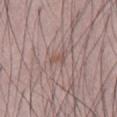Captured during whole-body skin photography for melanoma surveillance; the lesion was not biopsied. Imaged with white-light lighting. From the abdomen. The subject is a male about 50 years old. Approximately 2.5 mm at its widest. A 15 mm close-up extracted from a 3D total-body photography capture.